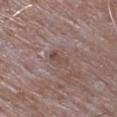notes = imaged on a skin check; not biopsied | tile lighting = white-light illumination | anatomic site = the left upper arm | image source = total-body-photography crop, ~15 mm field of view | size = about 3 mm | patient = male, in their mid-60s.